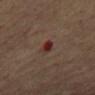{"biopsy_status": "not biopsied; imaged during a skin examination", "image": {"source": "total-body photography crop", "field_of_view_mm": 15}, "automated_metrics": {"area_mm2_approx": 3.0, "shape_asymmetry": 0.3}, "lighting": "cross-polarized", "site": "mid back", "lesion_size": {"long_diameter_mm_approx": 2.5}, "patient": {"sex": "male", "age_approx": 65}}